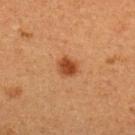biopsy status = total-body-photography surveillance lesion; no biopsy
location = the upper back
image source = ~15 mm tile from a whole-body skin photo
subject = female, aged 38–42
lighting = cross-polarized
size = ≈2.5 mm